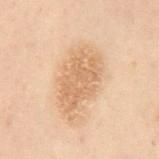Recorded during total-body skin imaging; not selected for excision or biopsy.
A lesion tile, about 15 mm wide, cut from a 3D total-body photograph.
The patient is a male aged 48–52.
The lesion is located on the mid back.
This is a cross-polarized tile.
Longest diameter approximately 7 mm.
Automated image analysis of the tile measured a lesion area of about 21 mm² and a shape eccentricity near 0.8. It also reported a lesion–skin lightness drop of about 8 and a lesion-to-skin contrast of about 6.5 (normalized; higher = more distinct). The analysis additionally found a border-irregularity rating of about 3.5/10, a color-variation rating of about 2.5/10, and peripheral color asymmetry of about 1. It also reported a nevus-likeness score of about 5/100.